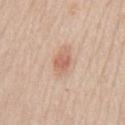| feature | finding |
|---|---|
| workup | imaged on a skin check; not biopsied |
| diameter | about 3.5 mm |
| location | the chest |
| patient | male, aged around 60 |
| acquisition | total-body-photography crop, ~15 mm field of view |
| lighting | white-light |